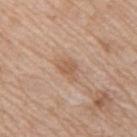Imaged during a routine full-body skin examination; the lesion was not biopsied and no histopathology is available. A female subject in their 40s. Measured at roughly 3 mm in maximum diameter. A 15 mm crop from a total-body photograph taken for skin-cancer surveillance. From the right upper arm. Captured under white-light illumination.